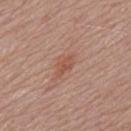This lesion was catalogued during total-body skin photography and was not selected for biopsy.
The lesion is located on the upper back.
A close-up tile cropped from a whole-body skin photograph, about 15 mm across.
The total-body-photography lesion software estimated an outline eccentricity of about 0.85 (0 = round, 1 = elongated) and two-axis asymmetry of about 0.3. And it measured a color-variation rating of about 1.5/10 and radial color variation of about 0.5.
About 3 mm across.
A male subject, in their mid-70s.
This is a white-light tile.